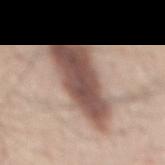No biopsy was performed on this lesion — it was imaged during a full skin examination and was not determined to be concerning. Cropped from a total-body skin-imaging series; the visible field is about 15 mm. The total-body-photography lesion software estimated a footprint of about 28 mm² and a shape-asymmetry score of about 0.3 (0 = symmetric). It also reported an average lesion color of about L≈52 a*≈18 b*≈23 (CIELAB), roughly 18 lightness units darker than nearby skin, and a lesion-to-skin contrast of about 12 (normalized; higher = more distinct). Captured under white-light illumination. On the mid back. The recorded lesion diameter is about 9.5 mm. A male patient, approximately 60 years of age.